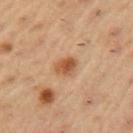Part of a total-body skin-imaging series; this lesion was reviewed on a skin check and was not flagged for biopsy. The recorded lesion diameter is about 3 mm. A lesion tile, about 15 mm wide, cut from a 3D total-body photograph. A male subject, in their mid- to late 50s. This is a cross-polarized tile. On the arm.